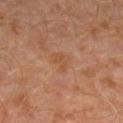The lesion was photographed on a routine skin check and not biopsied; there is no pathology result.
The lesion is located on the left leg.
Measured at roughly 2.5 mm in maximum diameter.
Imaged with cross-polarized lighting.
A 15 mm close-up tile from a total-body photography series done for melanoma screening.
Automated image analysis of the tile measured a footprint of about 4 mm², an eccentricity of roughly 0.6, and a shape-asymmetry score of about 0.5 (0 = symmetric).
A male subject, approximately 30 years of age.